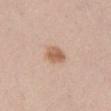Q: Is there a histopathology result?
A: total-body-photography surveillance lesion; no biopsy
Q: What kind of image is this?
A: ~15 mm crop, total-body skin-cancer survey
Q: Where on the body is the lesion?
A: the abdomen
Q: Patient demographics?
A: male, aged 58 to 62